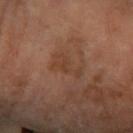Recorded during total-body skin imaging; not selected for excision or biopsy.
From the right forearm.
Cropped from a whole-body photographic skin survey; the tile spans about 15 mm.
An algorithmic analysis of the crop reported a lesion area of about 5.5 mm², a shape eccentricity near 0.85, and two-axis asymmetry of about 0.55. The analysis additionally found a lesion color around L≈41 a*≈20 b*≈30 in CIELAB and a normalized border contrast of about 5. The software also gave a classifier nevus-likeness of about 0/100 and a lesion-detection confidence of about 100/100.
Measured at roughly 3.5 mm in maximum diameter.
This is a cross-polarized tile.
A female subject aged approximately 70.